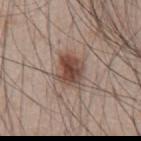<lesion>
  <biopsy_status>not biopsied; imaged during a skin examination</biopsy_status>
  <lesion_size>
    <long_diameter_mm_approx>3.5</long_diameter_mm_approx>
  </lesion_size>
  <site>front of the torso</site>
  <automated_metrics>
    <area_mm2_approx>9.0</area_mm2_approx>
    <eccentricity>0.6</eccentricity>
    <shape_asymmetry>0.3</shape_asymmetry>
    <nevus_likeness_0_100>95</nevus_likeness_0_100>
    <lesion_detection_confidence_0_100>100</lesion_detection_confidence_0_100>
  </automated_metrics>
  <image>
    <source>total-body photography crop</source>
    <field_of_view_mm>15</field_of_view_mm>
  </image>
  <patient>
    <sex>male</sex>
    <age_approx>45</age_approx>
  </patient>
</lesion>